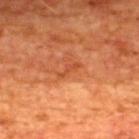{
  "biopsy_status": "not biopsied; imaged during a skin examination"
}